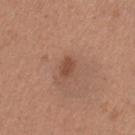Imaged during a routine full-body skin examination; the lesion was not biopsied and no histopathology is available.
Automated image analysis of the tile measured a lesion area of about 3 mm² and a shape eccentricity near 0.8. The software also gave an average lesion color of about L≈47 a*≈22 b*≈29 (CIELAB), roughly 9 lightness units darker than nearby skin, and a normalized border contrast of about 7.
On the left thigh.
A female subject, roughly 40 years of age.
The tile uses white-light illumination.
This image is a 15 mm lesion crop taken from a total-body photograph.
The lesion's longest dimension is about 2.5 mm.